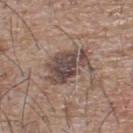notes: catalogued during a skin exam; not biopsied
site: the upper back
illumination: white-light
image: ~15 mm crop, total-body skin-cancer survey
lesion size: about 5.5 mm
subject: male, aged approximately 65
automated lesion analysis: a border-irregularity index near 4.5/10, internal color variation of about 4.5 on a 0–10 scale, and radial color variation of about 1.5